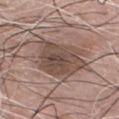Impression: The lesion was tiled from a total-body skin photograph and was not biopsied. Acquisition and patient details: Captured under white-light illumination. Automated image analysis of the tile measured a mean CIELAB color near L≈47 a*≈15 b*≈23, about 11 CIELAB-L* units darker than the surrounding skin, and a normalized lesion–skin contrast near 8. The software also gave a border-irregularity rating of about 2.5/10 and a peripheral color-asymmetry measure near 1.5. A male patient, approximately 75 years of age. Approximately 6 mm at its widest. The lesion is on the chest. Cropped from a total-body skin-imaging series; the visible field is about 15 mm.